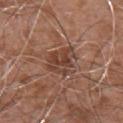| feature | finding |
|---|---|
| notes | total-body-photography surveillance lesion; no biopsy |
| image source | total-body-photography crop, ~15 mm field of view |
| subject | male, aged around 75 |
| anatomic site | the front of the torso |